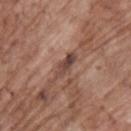{"biopsy_status": "not biopsied; imaged during a skin examination", "lesion_size": {"long_diameter_mm_approx": 3.5}, "site": "upper back", "image": {"source": "total-body photography crop", "field_of_view_mm": 15}, "automated_metrics": {"cielab_L": 44, "cielab_a": 20, "cielab_b": 24, "vs_skin_contrast_norm": 9.0, "border_irregularity_0_10": 4.0, "peripheral_color_asymmetry": 2.0, "lesion_detection_confidence_0_100": 90}, "patient": {"sex": "male", "age_approx": 70}, "lighting": "white-light"}